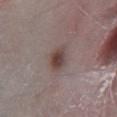| feature | finding |
|---|---|
| biopsy status | catalogued during a skin exam; not biopsied |
| automated metrics | an area of roughly 6 mm², an eccentricity of roughly 0.7, and two-axis asymmetry of about 0.15; a lesion color around L≈43 a*≈15 b*≈18 in CIELAB, a lesion–skin lightness drop of about 10, and a lesion-to-skin contrast of about 8.5 (normalized; higher = more distinct); border irregularity of about 2 on a 0–10 scale, internal color variation of about 4.5 on a 0–10 scale, and radial color variation of about 1.5 |
| image source | ~15 mm crop, total-body skin-cancer survey |
| site | the right lower leg |
| subject | male, aged 63 to 67 |
| size | ~3.5 mm (longest diameter) |